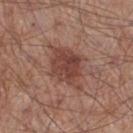follow-up — catalogued during a skin exam; not biopsied | automated lesion analysis — a footprint of about 14 mm²; a border-irregularity rating of about 3.5/10, internal color variation of about 3.5 on a 0–10 scale, and peripheral color asymmetry of about 1; a nevus-likeness score of about 75/100 and a lesion-detection confidence of about 100/100 | site — the leg | subject — male, aged 53 to 57 | image — ~15 mm tile from a whole-body skin photo | lesion size — ≈6 mm.